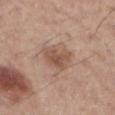The lesion was photographed on a routine skin check and not biopsied; there is no pathology result.